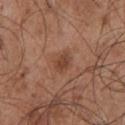This lesion was catalogued during total-body skin photography and was not selected for biopsy. Cropped from a total-body skin-imaging series; the visible field is about 15 mm. From the chest. A male subject about 55 years old.